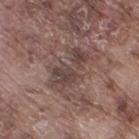Impression:
The lesion was photographed on a routine skin check and not biopsied; there is no pathology result.
Acquisition and patient details:
The tile uses white-light illumination. Cropped from a total-body skin-imaging series; the visible field is about 15 mm. The lesion-visualizer software estimated a lesion area of about 12 mm², an outline eccentricity of about 0.85 (0 = round, 1 = elongated), and a symmetry-axis asymmetry near 0.35. The software also gave an average lesion color of about L≈42 a*≈16 b*≈19 (CIELAB), about 8 CIELAB-L* units darker than the surrounding skin, and a normalized border contrast of about 7. The analysis additionally found a within-lesion color-variation index near 5/10 and radial color variation of about 2. The subject is a male aged around 75. Located on the right thigh.